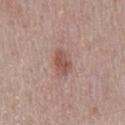Imaged during a routine full-body skin examination; the lesion was not biopsied and no histopathology is available. Automated tile analysis of the lesion measured a within-lesion color-variation index near 2/10 and peripheral color asymmetry of about 0.5. The software also gave a lesion-detection confidence of about 100/100. The lesion is on the mid back. A male patient, roughly 70 years of age. Approximately 3.5 mm at its widest. A lesion tile, about 15 mm wide, cut from a 3D total-body photograph. Captured under white-light illumination.